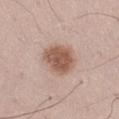Assessment: Imaged during a routine full-body skin examination; the lesion was not biopsied and no histopathology is available. Image and clinical context: The lesion is located on the right thigh. Measured at roughly 4.5 mm in maximum diameter. The subject is a male aged around 50. A close-up tile cropped from a whole-body skin photograph, about 15 mm across. Automated image analysis of the tile measured an area of roughly 13 mm², an outline eccentricity of about 0.7 (0 = round, 1 = elongated), and two-axis asymmetry of about 0.15. And it measured a lesion color around L≈56 a*≈19 b*≈27 in CIELAB, about 13 CIELAB-L* units darker than the surrounding skin, and a normalized lesion–skin contrast near 9. The analysis additionally found a classifier nevus-likeness of about 95/100.